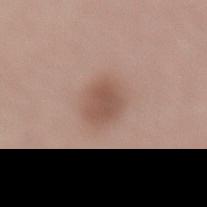Impression:
The lesion was tiled from a total-body skin photograph and was not biopsied.
Acquisition and patient details:
Located on the lower back. A lesion tile, about 15 mm wide, cut from a 3D total-body photograph. The patient is a male approximately 55 years of age.